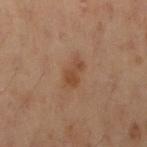* biopsy status — catalogued during a skin exam; not biopsied
* diameter — ≈3 mm
* image-analysis metrics — a lesion color around L≈37 a*≈17 b*≈27 in CIELAB and a normalized border contrast of about 6.5; a classifier nevus-likeness of about 20/100 and lesion-presence confidence of about 100/100
* tile lighting — cross-polarized
* anatomic site — the left arm
* subject — female, in their mid-50s
* image source — ~15 mm tile from a whole-body skin photo